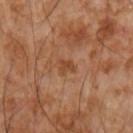Assessment:
The lesion was tiled from a total-body skin photograph and was not biopsied.
Acquisition and patient details:
Captured under cross-polarized illumination. From the arm. A male patient aged 53 to 57. This image is a 15 mm lesion crop taken from a total-body photograph.